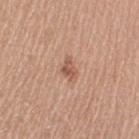workup = no biopsy performed (imaged during a skin exam); diameter = about 3 mm; subject = female, aged approximately 65; illumination = white-light; location = the left upper arm; image = 15 mm crop, total-body photography.